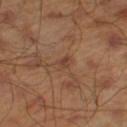workup = catalogued during a skin exam; not biopsied
body site = the left lower leg
patient = male, in their mid-40s
acquisition = ~15 mm tile from a whole-body skin photo